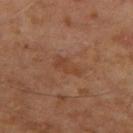Captured during whole-body skin photography for melanoma surveillance; the lesion was not biopsied. Cropped from a whole-body photographic skin survey; the tile spans about 15 mm. Imaged with cross-polarized lighting. Longest diameter approximately 3.5 mm. A male patient approximately 65 years of age. The lesion-visualizer software estimated a footprint of about 5 mm², an eccentricity of roughly 0.9, and a symmetry-axis asymmetry near 0.35. It also reported a lesion color around L≈41 a*≈21 b*≈30 in CIELAB, about 6 CIELAB-L* units darker than the surrounding skin, and a normalized border contrast of about 5.